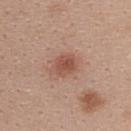biopsy status: catalogued during a skin exam; not biopsied
diameter: ~3 mm (longest diameter)
subject: female, in their mid- to late 20s
acquisition: ~15 mm crop, total-body skin-cancer survey
image-analysis metrics: an area of roughly 6 mm², an eccentricity of roughly 0.6, and a symmetry-axis asymmetry near 0.15; a lesion color around L≈52 a*≈24 b*≈28 in CIELAB, a lesion–skin lightness drop of about 10, and a normalized border contrast of about 7; border irregularity of about 1.5 on a 0–10 scale, internal color variation of about 3 on a 0–10 scale, and radial color variation of about 1
illumination: white-light
site: the upper back